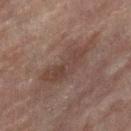Assessment:
This lesion was catalogued during total-body skin photography and was not selected for biopsy.
Clinical summary:
A female patient, aged 78 to 82. This image is a 15 mm lesion crop taken from a total-body photograph. Located on the leg. Approximately 8.5 mm at its widest. Imaged with cross-polarized lighting.